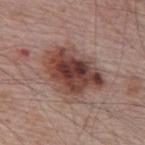The lesion was photographed on a routine skin check and not biopsied; there is no pathology result. A male subject, about 65 years old. Cropped from a whole-body photographic skin survey; the tile spans about 15 mm. The total-body-photography lesion software estimated a lesion area of about 26 mm² and two-axis asymmetry of about 0.3. The software also gave a border-irregularity index near 3.5/10, internal color variation of about 9 on a 0–10 scale, and radial color variation of about 3. From the upper back.